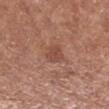notes=imaged on a skin check; not biopsied | location=the right forearm | acquisition=~15 mm tile from a whole-body skin photo | patient=female, aged around 50 | lesion size=about 3 mm | lighting=white-light illumination.